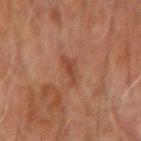Q: What did automated image analysis measure?
A: two-axis asymmetry of about 0.35; border irregularity of about 3.5 on a 0–10 scale, a color-variation rating of about 2.5/10, and peripheral color asymmetry of about 1
Q: How was the tile lit?
A: cross-polarized illumination
Q: What is the anatomic site?
A: the left forearm
Q: Patient demographics?
A: male, in their 60s
Q: How was this image acquired?
A: 15 mm crop, total-body photography
Q: Lesion size?
A: about 3 mm